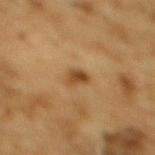biopsy status: total-body-photography surveillance lesion; no biopsy | subject: male, approximately 85 years of age or older | lesion size: ≈3 mm | acquisition: total-body-photography crop, ~15 mm field of view | TBP lesion metrics: a mean CIELAB color near L≈40 a*≈17 b*≈34, about 10 CIELAB-L* units darker than the surrounding skin, and a normalized border contrast of about 8.5; a border-irregularity rating of about 3/10, a within-lesion color-variation index near 4/10, and peripheral color asymmetry of about 1.5 | body site: the back.